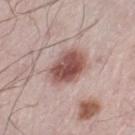Clinical impression: No biopsy was performed on this lesion — it was imaged during a full skin examination and was not determined to be concerning. Context: An algorithmic analysis of the crop reported a lesion area of about 12 mm². It also reported an average lesion color of about L≈51 a*≈22 b*≈23 (CIELAB), a lesion–skin lightness drop of about 16, and a lesion-to-skin contrast of about 11 (normalized; higher = more distinct). The analysis additionally found a classifier nevus-likeness of about 95/100 and lesion-presence confidence of about 100/100. The subject is a male in their mid-50s. Longest diameter approximately 4.5 mm. A lesion tile, about 15 mm wide, cut from a 3D total-body photograph. Located on the leg.